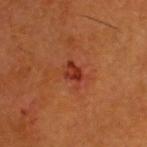<lesion>
<biopsy_status>not biopsied; imaged during a skin examination</biopsy_status>
<automated_metrics>
  <cielab_L>35</cielab_L>
  <cielab_a>31</cielab_a>
  <cielab_b>33</cielab_b>
  <vs_skin_darker_L>9.0</vs_skin_darker_L>
  <nevus_likeness_0_100>5</nevus_likeness_0_100>
  <lesion_detection_confidence_0_100>100</lesion_detection_confidence_0_100>
</automated_metrics>
<lesion_size>
  <long_diameter_mm_approx>3.0</long_diameter_mm_approx>
</lesion_size>
<image>
  <source>total-body photography crop</source>
  <field_of_view_mm>15</field_of_view_mm>
</image>
<lighting>cross-polarized</lighting>
<patient>
  <sex>male</sex>
  <age_approx>60</age_approx>
</patient>
<site>head or neck</site>
</lesion>